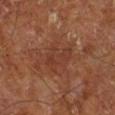Impression:
The lesion was tiled from a total-body skin photograph and was not biopsied.
Image and clinical context:
The subject is a male aged approximately 65. From the right lower leg. A roughly 15 mm field-of-view crop from a total-body skin photograph. Approximately 4 mm at its widest. Captured under cross-polarized illumination. The lesion-visualizer software estimated a mean CIELAB color near L≈35 a*≈22 b*≈28, about 5 CIELAB-L* units darker than the surrounding skin, and a normalized border contrast of about 5. And it measured an automated nevus-likeness rating near 0 out of 100.